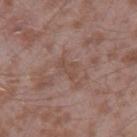{
  "biopsy_status": "not biopsied; imaged during a skin examination",
  "image": {
    "source": "total-body photography crop",
    "field_of_view_mm": 15
  },
  "site": "right thigh",
  "automated_metrics": {
    "eccentricity": 0.85,
    "shape_asymmetry": 0.55,
    "cielab_L": 48,
    "cielab_a": 18,
    "cielab_b": 24,
    "vs_skin_darker_L": 5.0,
    "vs_skin_contrast_norm": 4.5,
    "nevus_likeness_0_100": 0
  },
  "patient": {
    "sex": "male",
    "age_approx": 45
  },
  "lighting": "white-light",
  "lesion_size": {
    "long_diameter_mm_approx": 2.5
  }
}